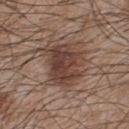The lesion was photographed on a routine skin check and not biopsied; there is no pathology result. Measured at roughly 5.5 mm in maximum diameter. Captured under white-light illumination. This image is a 15 mm lesion crop taken from a total-body photograph. The patient is a male aged 43–47. From the upper back. The total-body-photography lesion software estimated an area of roughly 19 mm². The analysis additionally found a border-irregularity index near 3.5/10, a within-lesion color-variation index near 5/10, and a peripheral color-asymmetry measure near 2. And it measured a classifier nevus-likeness of about 65/100.